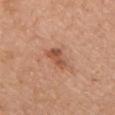Clinical impression:
No biopsy was performed on this lesion — it was imaged during a full skin examination and was not determined to be concerning.
Background:
Located on the chest. A male patient, in their mid-60s. The lesion-visualizer software estimated a footprint of about 4.5 mm², a shape eccentricity near 0.8, and a symmetry-axis asymmetry near 0.4. The software also gave a mean CIELAB color near L≈53 a*≈24 b*≈34 and roughly 11 lightness units darker than nearby skin. The software also gave a border-irregularity rating of about 4/10, a color-variation rating of about 3/10, and peripheral color asymmetry of about 1. And it measured an automated nevus-likeness rating near 15 out of 100 and a lesion-detection confidence of about 100/100. A 15 mm close-up tile from a total-body photography series done for melanoma screening.